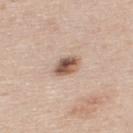workup — catalogued during a skin exam; not biopsied | imaging modality — total-body-photography crop, ~15 mm field of view | diameter — ≈3 mm | body site — the upper back | automated metrics — a footprint of about 5.5 mm²; a normalized border contrast of about 10.5; a border-irregularity rating of about 1.5/10, a within-lesion color-variation index near 7.5/10, and peripheral color asymmetry of about 3 | lighting — white-light | subject — male, aged approximately 45.